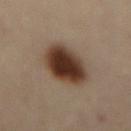notes — total-body-photography surveillance lesion; no biopsy | lighting — cross-polarized illumination | subject — female, in their 60s | imaging modality — ~15 mm crop, total-body skin-cancer survey | body site — the mid back | lesion size — ≈6 mm.